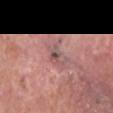Clinical impression: Imaged during a routine full-body skin examination; the lesion was not biopsied and no histopathology is available. Context: About 3 mm across. A female patient in their mid-80s or older. The lesion is on the head or neck. Cropped from a total-body skin-imaging series; the visible field is about 15 mm.